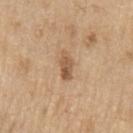<case>
  <biopsy_status>not biopsied; imaged during a skin examination</biopsy_status>
  <lighting>white-light</lighting>
  <patient>
    <sex>male</sex>
    <age_approx>70</age_approx>
  </patient>
  <site>left upper arm</site>
  <lesion_size>
    <long_diameter_mm_approx>3.5</long_diameter_mm_approx>
  </lesion_size>
  <image>
    <source>total-body photography crop</source>
    <field_of_view_mm>15</field_of_view_mm>
  </image>
</case>